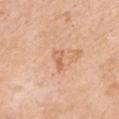  patient:
    sex: female
    age_approx: 55
  lighting: white-light
  image:
    source: total-body photography crop
    field_of_view_mm: 15
  lesion_size:
    long_diameter_mm_approx: 2.5
  site: chest